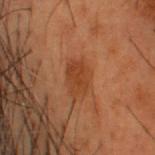<record>
  <biopsy_status>not biopsied; imaged during a skin examination</biopsy_status>
  <lighting>cross-polarized</lighting>
  <patient>
    <sex>male</sex>
    <age_approx>55</age_approx>
  </patient>
  <image>
    <source>total-body photography crop</source>
    <field_of_view_mm>15</field_of_view_mm>
  </image>
  <automated_metrics>
    <border_irregularity_0_10>2.5</border_irregularity_0_10>
    <color_variation_0_10>2.0</color_variation_0_10>
    <peripheral_color_asymmetry>0.5</peripheral_color_asymmetry>
  </automated_metrics>
  <lesion_size>
    <long_diameter_mm_approx>3.5</long_diameter_mm_approx>
  </lesion_size>
  <site>head or neck</site>
</record>